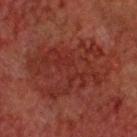workup: total-body-photography surveillance lesion; no biopsy | patient: male, in their 60s | anatomic site: the head or neck | tile lighting: cross-polarized | image: ~15 mm crop, total-body skin-cancer survey | diameter: about 9.5 mm.